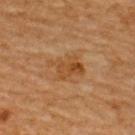Impression: The lesion was tiled from a total-body skin photograph and was not biopsied. Acquisition and patient details: The subject is a male roughly 65 years of age. Cropped from a total-body skin-imaging series; the visible field is about 15 mm. An algorithmic analysis of the crop reported an average lesion color of about L≈41 a*≈19 b*≈35 (CIELAB) and a lesion–skin lightness drop of about 7. The lesion is on the upper back.